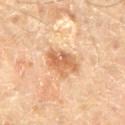{"biopsy_status": "not biopsied; imaged during a skin examination", "lesion_size": {"long_diameter_mm_approx": 4.5}, "site": "lower back", "patient": {"sex": "male", "age_approx": 65}, "image": {"source": "total-body photography crop", "field_of_view_mm": 15}}